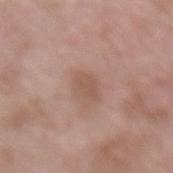workup: imaged on a skin check; not biopsied | image source: total-body-photography crop, ~15 mm field of view | lesion diameter: ≈3 mm | anatomic site: the lower back | automated metrics: a color-variation rating of about 1.5/10 and radial color variation of about 0.5 | tile lighting: white-light | subject: male, aged approximately 55.